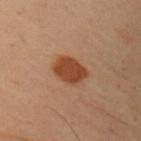No biopsy was performed on this lesion — it was imaged during a full skin examination and was not determined to be concerning. A 15 mm close-up tile from a total-body photography series done for melanoma screening. Approximately 3.5 mm at its widest. The lesion is located on the arm. An algorithmic analysis of the crop reported an area of roughly 8.5 mm², an eccentricity of roughly 0.65, and a shape-asymmetry score of about 0.2 (0 = symmetric). The analysis additionally found a mean CIELAB color near L≈35 a*≈20 b*≈29, a lesion–skin lightness drop of about 10, and a normalized border contrast of about 10. The analysis additionally found a border-irregularity index near 2/10, a color-variation rating of about 1.5/10, and peripheral color asymmetry of about 0.5. A male patient, aged around 55.